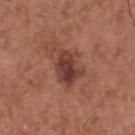body site: the head or neck; diameter: about 4 mm; subject: male, in their 50s; tile lighting: white-light; imaging modality: total-body-photography crop, ~15 mm field of view.